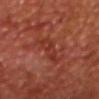notes: total-body-photography surveillance lesion; no biopsy
patient: male, in their mid-60s
illumination: cross-polarized illumination
image source: ~15 mm crop, total-body skin-cancer survey